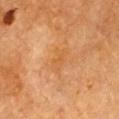Notes:
* biopsy status · total-body-photography surveillance lesion; no biopsy
* site · the chest
* illumination · cross-polarized illumination
* patient · male, aged approximately 85 or older
* imaging modality · ~15 mm tile from a whole-body skin photo
* size · ≈3.5 mm